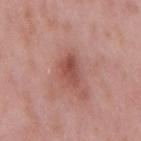The lesion was tiled from a total-body skin photograph and was not biopsied.
Automated image analysis of the tile measured a border-irregularity rating of about 3/10, a color-variation rating of about 4.5/10, and radial color variation of about 1.5.
Located on the mid back.
About 3.5 mm across.
A male patient aged around 55.
Imaged with white-light lighting.
A 15 mm close-up tile from a total-body photography series done for melanoma screening.